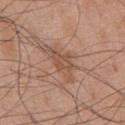From the chest. The lesion's longest dimension is about 5 mm. A male patient, aged around 75. A 15 mm close-up extracted from a 3D total-body photography capture.